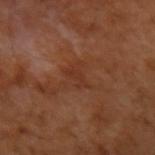biopsy_status: not biopsied; imaged during a skin examination
lesion_size:
  long_diameter_mm_approx: 3.0
automated_metrics:
  area_mm2_approx: 3.0
  eccentricity: 0.9
  shape_asymmetry: 0.4
  cielab_L: 34
  cielab_a: 24
  cielab_b: 30
  vs_skin_contrast_norm: 5.0
  border_irregularity_0_10: 5.0
  color_variation_0_10: 0.0
  peripheral_color_asymmetry: 0.0
  nevus_likeness_0_100: 0
patient:
  sex: male
  age_approx: 55
image:
  source: total-body photography crop
  field_of_view_mm: 15
site: left upper arm
lighting: cross-polarized